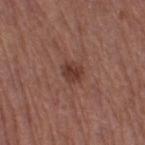Clinical summary:
Located on the right thigh. The lesion's longest dimension is about 2.5 mm. A female patient in their 50s. Captured under white-light illumination. A lesion tile, about 15 mm wide, cut from a 3D total-body photograph.